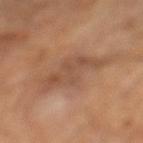Recorded during total-body skin imaging; not selected for excision or biopsy. This image is a 15 mm lesion crop taken from a total-body photograph. A male patient, aged approximately 65. Approximately 6.5 mm at its widest. This is a cross-polarized tile. The lesion is located on the left lower leg. The total-body-photography lesion software estimated an eccentricity of roughly 0.8 and a symmetry-axis asymmetry near 0.4. And it measured a border-irregularity index near 6/10, a within-lesion color-variation index near 3/10, and peripheral color asymmetry of about 1. The software also gave a classifier nevus-likeness of about 0/100 and lesion-presence confidence of about 100/100.